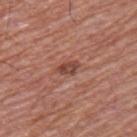This lesion was catalogued during total-body skin photography and was not selected for biopsy. On the arm. Cropped from a total-body skin-imaging series; the visible field is about 15 mm. A male patient, roughly 55 years of age. The lesion's longest dimension is about 3 mm. The total-body-photography lesion software estimated a footprint of about 3.5 mm², a shape eccentricity near 0.8, and two-axis asymmetry of about 0.3. And it measured a lesion color around L≈45 a*≈24 b*≈27 in CIELAB, about 11 CIELAB-L* units darker than the surrounding skin, and a normalized border contrast of about 8. And it measured a border-irregularity index near 3/10, a within-lesion color-variation index near 2.5/10, and a peripheral color-asymmetry measure near 1. And it measured lesion-presence confidence of about 100/100.